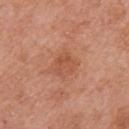Part of a total-body skin-imaging series; this lesion was reviewed on a skin check and was not flagged for biopsy. This image is a 15 mm lesion crop taken from a total-body photograph. The lesion is located on the right upper arm. Captured under white-light illumination. A female subject aged approximately 60. The recorded lesion diameter is about 2.5 mm.